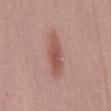biopsy_status: not biopsied; imaged during a skin examination
lesion_size:
  long_diameter_mm_approx: 5.5
patient:
  sex: female
  age_approx: 60
lighting: white-light
site: mid back
image:
  source: total-body photography crop
  field_of_view_mm: 15